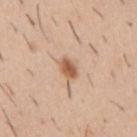Clinical impression:
Part of a total-body skin-imaging series; this lesion was reviewed on a skin check and was not flagged for biopsy.
Context:
The lesion is on the mid back. This is a white-light tile. Longest diameter approximately 2.5 mm. A 15 mm close-up tile from a total-body photography series done for melanoma screening. The lesion-visualizer software estimated a shape-asymmetry score of about 0.3 (0 = symmetric). It also reported a peripheral color-asymmetry measure near 1. A male subject, about 40 years old.